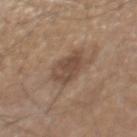Q: Is there a histopathology result?
A: no biopsy performed (imaged during a skin exam)
Q: What is the anatomic site?
A: the chest
Q: Illumination type?
A: white-light
Q: What is the lesion's diameter?
A: about 4 mm
Q: What kind of image is this?
A: ~15 mm tile from a whole-body skin photo
Q: Patient demographics?
A: male, aged around 70